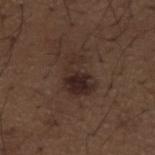| feature | finding |
|---|---|
| notes | catalogued during a skin exam; not biopsied |